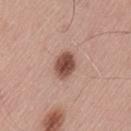Q: Was this lesion biopsied?
A: no biopsy performed (imaged during a skin exam)
Q: How was the tile lit?
A: white-light
Q: What is the lesion's diameter?
A: ~3 mm (longest diameter)
Q: What kind of image is this?
A: total-body-photography crop, ~15 mm field of view
Q: Lesion location?
A: the lower back
Q: What are the patient's age and sex?
A: male, aged 53 to 57